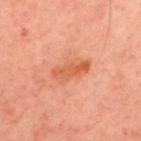This lesion was catalogued during total-body skin photography and was not selected for biopsy.